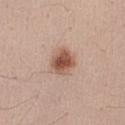No biopsy was performed on this lesion — it was imaged during a full skin examination and was not determined to be concerning.
The lesion-visualizer software estimated an area of roughly 7.5 mm² and a shape-asymmetry score of about 0.15 (0 = symmetric).
The lesion is on the front of the torso.
The subject is a male roughly 65 years of age.
Captured under white-light illumination.
Longest diameter approximately 3.5 mm.
Cropped from a total-body skin-imaging series; the visible field is about 15 mm.